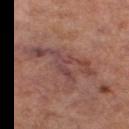A roughly 15 mm field-of-view crop from a total-body skin photograph. The lesion-visualizer software estimated border irregularity of about 10 on a 0–10 scale, internal color variation of about 4.5 on a 0–10 scale, and peripheral color asymmetry of about 1.5. The software also gave a nevus-likeness score of about 0/100 and a lesion-detection confidence of about 55/100. From the right thigh. Longest diameter approximately 7.5 mm. The tile uses cross-polarized illumination. A female subject aged around 60.